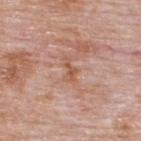Case summary:
- location: the upper back
- TBP lesion metrics: a lesion area of about 2.5 mm² and a shape-asymmetry score of about 0.45 (0 = symmetric); a mean CIELAB color near L≈55 a*≈22 b*≈31 and a normalized lesion–skin contrast near 6.5; an automated nevus-likeness rating near 0 out of 100 and lesion-presence confidence of about 100/100
- image: ~15 mm tile from a whole-body skin photo
- lighting: white-light
- size: ≈3 mm
- subject: male, about 75 years old